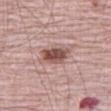Part of a total-body skin-imaging series; this lesion was reviewed on a skin check and was not flagged for biopsy.